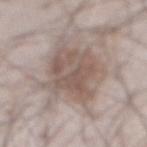The lesion is on the abdomen. Imaged with white-light lighting. A region of skin cropped from a whole-body photographic capture, roughly 15 mm wide. Approximately 6.5 mm at its widest. A male subject, aged 68 to 72.